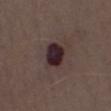Q: Is there a histopathology result?
A: total-body-photography surveillance lesion; no biopsy
Q: How large is the lesion?
A: about 3.5 mm
Q: Who is the patient?
A: male, aged approximately 70
Q: What is the anatomic site?
A: the chest
Q: What lighting was used for the tile?
A: white-light
Q: What is the imaging modality?
A: total-body-photography crop, ~15 mm field of view